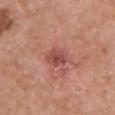Imaged during a routine full-body skin examination; the lesion was not biopsied and no histopathology is available.